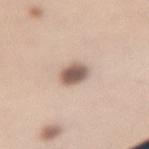| feature | finding |
|---|---|
| workup | catalogued during a skin exam; not biopsied |
| lesion diameter | about 3 mm |
| anatomic site | the right upper arm |
| acquisition | ~15 mm crop, total-body skin-cancer survey |
| lighting | white-light |
| subject | female, aged approximately 30 |